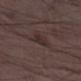workup: catalogued during a skin exam; not biopsied | subject: male, aged approximately 50 | illumination: white-light illumination | image: 15 mm crop, total-body photography | lesion diameter: ≈3 mm | site: the right lower leg | automated metrics: a shape eccentricity near 0.75 and a shape-asymmetry score of about 0.25 (0 = symmetric); a mean CIELAB color near L≈28 a*≈13 b*≈16, about 6 CIELAB-L* units darker than the surrounding skin, and a lesion-to-skin contrast of about 6.5 (normalized; higher = more distinct); internal color variation of about 1.5 on a 0–10 scale and radial color variation of about 0.5.